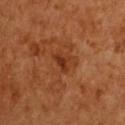Assessment:
Captured during whole-body skin photography for melanoma surveillance; the lesion was not biopsied.
Image and clinical context:
A female subject aged 53 to 57. A 15 mm close-up tile from a total-body photography series done for melanoma screening. An algorithmic analysis of the crop reported a border-irregularity rating of about 4.5/10, internal color variation of about 0.5 on a 0–10 scale, and peripheral color asymmetry of about 0. It also reported a nevus-likeness score of about 0/100. The lesion is located on the chest. The lesion's longest dimension is about 2.5 mm.